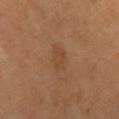biopsy_status: not biopsied; imaged during a skin examination
lesion_size:
  long_diameter_mm_approx: 3.0
patient:
  age_approx: 60
image:
  source: total-body photography crop
  field_of_view_mm: 15
lighting: cross-polarized
site: right upper arm